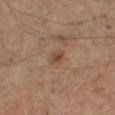Captured during whole-body skin photography for melanoma surveillance; the lesion was not biopsied.
The lesion-visualizer software estimated a footprint of about 2.5 mm², an eccentricity of roughly 0.7, and a symmetry-axis asymmetry near 0.25. And it measured a lesion color around L≈43 a*≈18 b*≈29 in CIELAB and about 8 CIELAB-L* units darker than the surrounding skin. And it measured an automated nevus-likeness rating near 20 out of 100.
The lesion's longest dimension is about 2 mm.
A close-up tile cropped from a whole-body skin photograph, about 15 mm across.
The lesion is on the left lower leg.
A male patient roughly 55 years of age.